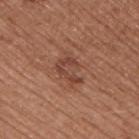biopsy_status: not biopsied; imaged during a skin examination
lesion_size:
  long_diameter_mm_approx: 4.0
image:
  source: total-body photography crop
  field_of_view_mm: 15
patient:
  sex: male
  age_approx: 55
site: right upper arm
lighting: white-light
automated_metrics:
  area_mm2_approx: 8.0
  eccentricity: 0.75
  shape_asymmetry: 0.3
  vs_skin_darker_L: 8.0
  vs_skin_contrast_norm: 6.0
  border_irregularity_0_10: 4.0
  color_variation_0_10: 4.0
  nevus_likeness_0_100: 0